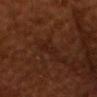{"biopsy_status": "not biopsied; imaged during a skin examination", "patient": {"sex": "male", "age_approx": 65}, "lighting": "cross-polarized", "site": "head or neck", "automated_metrics": {"area_mm2_approx": 4.0, "eccentricity": 0.9, "shape_asymmetry": 0.5, "cielab_L": 20, "cielab_a": 20, "cielab_b": 24, "vs_skin_darker_L": 4.0, "vs_skin_contrast_norm": 5.0, "border_irregularity_0_10": 5.5, "peripheral_color_asymmetry": 0.0, "nevus_likeness_0_100": 0, "lesion_detection_confidence_0_100": 75}, "image": {"source": "total-body photography crop", "field_of_view_mm": 15}, "lesion_size": {"long_diameter_mm_approx": 3.5}}